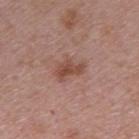{
  "biopsy_status": "not biopsied; imaged during a skin examination",
  "site": "upper back",
  "image": {
    "source": "total-body photography crop",
    "field_of_view_mm": 15
  },
  "patient": {
    "sex": "female",
    "age_approx": 45
  }
}